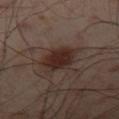illumination = cross-polarized; lesion size = ≈4.5 mm; body site = the leg; subject = male, in their mid-50s; imaging modality = ~15 mm crop, total-body skin-cancer survey.